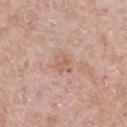Assessment:
This lesion was catalogued during total-body skin photography and was not selected for biopsy.
Acquisition and patient details:
A 15 mm crop from a total-body photograph taken for skin-cancer surveillance. Captured under white-light illumination. A male patient, aged 58 to 62. Measured at roughly 2.5 mm in maximum diameter. The total-body-photography lesion software estimated a lesion area of about 3.5 mm², a shape eccentricity near 0.7, and two-axis asymmetry of about 0.25. It also reported a border-irregularity index near 2.5/10, a within-lesion color-variation index near 2/10, and peripheral color asymmetry of about 0.5. On the abdomen.